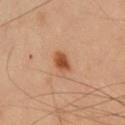Image and clinical context:
A male patient, aged 53 to 57. The total-body-photography lesion software estimated an area of roughly 4 mm², a shape eccentricity near 0.65, and a symmetry-axis asymmetry near 0.25. Imaged with cross-polarized lighting. Approximately 2.5 mm at its widest. Cropped from a whole-body photographic skin survey; the tile spans about 15 mm. Located on the chest.